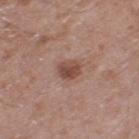Imaged during a routine full-body skin examination; the lesion was not biopsied and no histopathology is available. The tile uses white-light illumination. Measured at roughly 3 mm in maximum diameter. Cropped from a whole-body photographic skin survey; the tile spans about 15 mm. On the right thigh. The subject is a male aged 58–62.